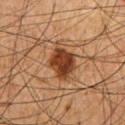<tbp_lesion>
  <biopsy_status>not biopsied; imaged during a skin examination</biopsy_status>
</tbp_lesion>